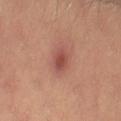Recorded during total-body skin imaging; not selected for excision or biopsy. The patient is a female aged 33–37. This is a cross-polarized tile. A region of skin cropped from a whole-body photographic capture, roughly 15 mm wide. The lesion is located on the right thigh.